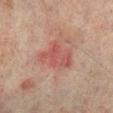<record>
<biopsy_status>not biopsied; imaged during a skin examination</biopsy_status>
<site>right lower leg</site>
<lesion_size>
  <long_diameter_mm_approx>5.0</long_diameter_mm_approx>
</lesion_size>
<image>
  <source>total-body photography crop</source>
  <field_of_view_mm>15</field_of_view_mm>
</image>
<patient>
  <sex>male</sex>
  <age_approx>75</age_approx>
</patient>
<lighting>cross-polarized</lighting>
<automated_metrics>
  <vs_skin_darker_L>7.0</vs_skin_darker_L>
  <vs_skin_contrast_norm>6.0</vs_skin_contrast_norm>
  <color_variation_0_10>3.0</color_variation_0_10>
  <nevus_likeness_0_100>0</nevus_likeness_0_100>
</automated_metrics>
</record>